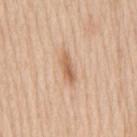Assessment:
Recorded during total-body skin imaging; not selected for excision or biopsy.
Acquisition and patient details:
A 15 mm crop from a total-body photograph taken for skin-cancer surveillance. The lesion is located on the mid back. A male subject aged around 60. Captured under white-light illumination. The recorded lesion diameter is about 4 mm.